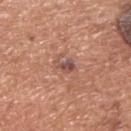workup: no biopsy performed (imaged during a skin exam) | lesion diameter: about 2.5 mm | acquisition: ~15 mm tile from a whole-body skin photo | lighting: white-light illumination | automated lesion analysis: a footprint of about 3.5 mm², an outline eccentricity of about 0.65 (0 = round, 1 = elongated), and two-axis asymmetry of about 0.4; a detector confidence of about 100 out of 100 that the crop contains a lesion | patient: male, aged around 65 | location: the upper back.